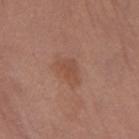Captured during whole-body skin photography for melanoma surveillance; the lesion was not biopsied. Located on the leg. Imaged with white-light lighting. A female subject, aged approximately 65. Automated image analysis of the tile measured an area of roughly 4 mm², an eccentricity of roughly 0.75, and two-axis asymmetry of about 0.35. The analysis additionally found roughly 7 lightness units darker than nearby skin and a normalized lesion–skin contrast near 5.5. The analysis additionally found a border-irregularity index near 3.5/10 and peripheral color asymmetry of about 0.5. And it measured a classifier nevus-likeness of about 5/100 and lesion-presence confidence of about 100/100. Measured at roughly 3 mm in maximum diameter. Cropped from a total-body skin-imaging series; the visible field is about 15 mm.